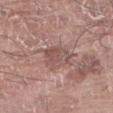This lesion was catalogued during total-body skin photography and was not selected for biopsy. Captured under white-light illumination. Cropped from a total-body skin-imaging series; the visible field is about 15 mm. A male subject aged approximately 75. The lesion is located on the left forearm.